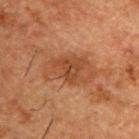Q: Is there a histopathology result?
A: imaged on a skin check; not biopsied
Q: What is the lesion's diameter?
A: ≈5.5 mm
Q: What is the anatomic site?
A: the upper back
Q: Illumination type?
A: cross-polarized
Q: How was this image acquired?
A: 15 mm crop, total-body photography
Q: What are the patient's age and sex?
A: male, approximately 50 years of age
Q: Automated lesion metrics?
A: a footprint of about 15 mm² and an eccentricity of roughly 0.8; a color-variation rating of about 3.5/10